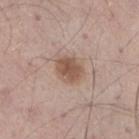Impression:
The lesion was tiled from a total-body skin photograph and was not biopsied.
Context:
The tile uses white-light illumination. The lesion is located on the right thigh. A 15 mm close-up tile from a total-body photography series done for melanoma screening. Longest diameter approximately 3.5 mm. A male subject, aged 53–57.